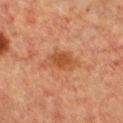This lesion was catalogued during total-body skin photography and was not selected for biopsy.
Captured under cross-polarized illumination.
The lesion's longest dimension is about 4.5 mm.
A roughly 15 mm field-of-view crop from a total-body skin photograph.
A female patient, in their mid- to late 50s.
The lesion is on the chest.